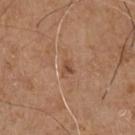notes=catalogued during a skin exam; not biopsied | subject=male, in their 70s | imaging modality=~15 mm crop, total-body skin-cancer survey | body site=the chest | lighting=white-light illumination | lesion size=~2.5 mm (longest diameter).